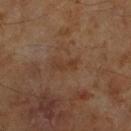Clinical impression: Imaged during a routine full-body skin examination; the lesion was not biopsied and no histopathology is available. Image and clinical context: This is a cross-polarized tile. The recorded lesion diameter is about 3 mm. A close-up tile cropped from a whole-body skin photograph, about 15 mm across. The lesion is located on the leg. Automated tile analysis of the lesion measured a mean CIELAB color near L≈33 a*≈16 b*≈27 and a lesion-to-skin contrast of about 5.5 (normalized; higher = more distinct). The analysis additionally found a lesion-detection confidence of about 100/100. A male subject aged 58–62.